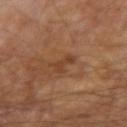biopsy_status: not biopsied; imaged during a skin examination
image:
  source: total-body photography crop
  field_of_view_mm: 15
patient:
  sex: male
  age_approx: 65
site: left arm